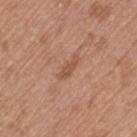Part of a total-body skin-imaging series; this lesion was reviewed on a skin check and was not flagged for biopsy.
A 15 mm close-up extracted from a 3D total-body photography capture.
Located on the left thigh.
A female subject in their mid-70s.